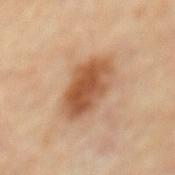• notes: no biopsy performed (imaged during a skin exam)
• tile lighting: cross-polarized
• imaging modality: ~15 mm crop, total-body skin-cancer survey
• automated lesion analysis: an area of roughly 16 mm² and a shape-asymmetry score of about 0.1 (0 = symmetric); a classifier nevus-likeness of about 95/100 and a detector confidence of about 100 out of 100 that the crop contains a lesion
• anatomic site: the mid back
• subject: male, in their mid- to late 80s or older
• diameter: ≈6 mm